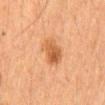notes = catalogued during a skin exam; not biopsied
lesion diameter = about 4.5 mm
image = 15 mm crop, total-body photography
image-analysis metrics = a lesion color around L≈48 a*≈22 b*≈35 in CIELAB, a lesion–skin lightness drop of about 10, and a normalized border contrast of about 7.5
subject = male, aged 63–67
body site = the mid back